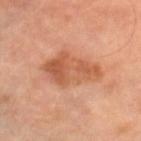biopsy status: catalogued during a skin exam; not biopsied
lighting: cross-polarized illumination
automated metrics: an area of roughly 17 mm², an outline eccentricity of about 0.8 (0 = round, 1 = elongated), and two-axis asymmetry of about 0.3; a classifier nevus-likeness of about 5/100 and a detector confidence of about 100 out of 100 that the crop contains a lesion
image: total-body-photography crop, ~15 mm field of view
anatomic site: the left leg
diameter: ~6 mm (longest diameter)
subject: female, in their 60s